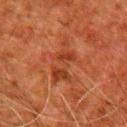Assessment: This lesion was catalogued during total-body skin photography and was not selected for biopsy. Image and clinical context: A 15 mm crop from a total-body photograph taken for skin-cancer surveillance. A male patient aged 78–82. The lesion is on the right upper arm. Captured under cross-polarized illumination. Approximately 6 mm at its widest.